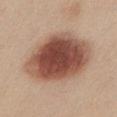The lesion was photographed on a routine skin check and not biopsied; there is no pathology result. A female patient aged approximately 40. The lesion is on the chest. Cropped from a total-body skin-imaging series; the visible field is about 15 mm.